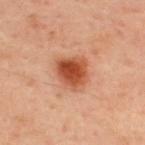Imaged during a routine full-body skin examination; the lesion was not biopsied and no histopathology is available. From the upper back. Approximately 3.5 mm at its widest. This is a cross-polarized tile. The patient is a male in their mid-40s. Automated image analysis of the tile measured an average lesion color of about L≈51 a*≈30 b*≈37 (CIELAB), a lesion–skin lightness drop of about 14, and a lesion-to-skin contrast of about 10 (normalized; higher = more distinct). The analysis additionally found a border-irregularity rating of about 2/10, a color-variation rating of about 6.5/10, and peripheral color asymmetry of about 2. The software also gave a nevus-likeness score of about 100/100 and a lesion-detection confidence of about 100/100. A lesion tile, about 15 mm wide, cut from a 3D total-body photograph.